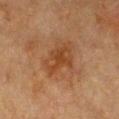{"biopsy_status": "not biopsied; imaged during a skin examination", "automated_metrics": {"cielab_L": 36, "cielab_a": 20, "cielab_b": 31, "vs_skin_contrast_norm": 6.5}, "image": {"source": "total-body photography crop", "field_of_view_mm": 15}, "site": "chest", "lesion_size": {"long_diameter_mm_approx": 4.0}, "patient": {"sex": "female", "age_approx": 55}}